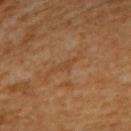biopsy status — imaged on a skin check; not biopsied | image source — ~15 mm tile from a whole-body skin photo | patient — female, aged around 65 | lighting — cross-polarized | anatomic site — the upper back | automated lesion analysis — an eccentricity of roughly 0.95 and a shape-asymmetry score of about 0.45 (0 = symmetric) | lesion size — ~3 mm (longest diameter).